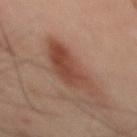Assessment: No biopsy was performed on this lesion — it was imaged during a full skin examination and was not determined to be concerning. Image and clinical context: Cropped from a total-body skin-imaging series; the visible field is about 15 mm. The patient is a male aged 48 to 52. The lesion is on the mid back.